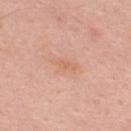Cropped from a total-body skin-imaging series; the visible field is about 15 mm.
Measured at roughly 3 mm in maximum diameter.
The lesion is located on the upper back.
The patient is a male aged around 65.